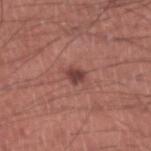Impression: This lesion was catalogued during total-body skin photography and was not selected for biopsy. Clinical summary: The lesion is on the left lower leg. The subject is a male roughly 45 years of age. Longest diameter approximately 2.5 mm. A roughly 15 mm field-of-view crop from a total-body skin photograph.